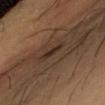Findings:
– follow-up: no biopsy performed (imaged during a skin exam)
– tile lighting: cross-polarized illumination
– patient: female, aged 48 to 52
– image source: 15 mm crop, total-body photography